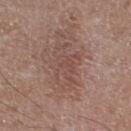| feature | finding |
|---|---|
| follow-up | imaged on a skin check; not biopsied |
| diameter | ≈5.5 mm |
| image source | 15 mm crop, total-body photography |
| subject | male, about 50 years old |
| illumination | white-light illumination |
| automated lesion analysis | an area of roughly 12 mm²; a lesion-to-skin contrast of about 5 (normalized; higher = more distinct); a border-irregularity rating of about 6.5/10 and peripheral color asymmetry of about 1 |
| anatomic site | the left lower leg |